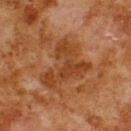Image and clinical context:
Longest diameter approximately 6.5 mm. Captured under cross-polarized illumination. From the back. A roughly 15 mm field-of-view crop from a total-body skin photograph. Automated tile analysis of the lesion measured a lesion area of about 15 mm², a shape eccentricity near 0.75, and a symmetry-axis asymmetry near 0.7. The software also gave a color-variation rating of about 3/10 and radial color variation of about 1. The software also gave an automated nevus-likeness rating near 0 out of 100 and lesion-presence confidence of about 100/100. The patient is a male about 80 years old.